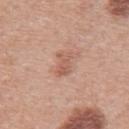Longest diameter approximately 3 mm. This image is a 15 mm lesion crop taken from a total-body photograph. Imaged with white-light lighting. On the mid back. A male patient, aged 68–72.